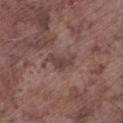This lesion was catalogued during total-body skin photography and was not selected for biopsy.
Located on the leg.
A close-up tile cropped from a whole-body skin photograph, about 15 mm across.
The patient is a male aged approximately 75.
This is a white-light tile.